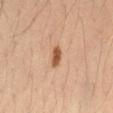workup: total-body-photography surveillance lesion; no biopsy | patient: male, aged around 35 | anatomic site: the lower back | imaging modality: 15 mm crop, total-body photography | size: ~3 mm (longest diameter) | illumination: cross-polarized | TBP lesion metrics: a lesion area of about 4 mm²; a lesion color around L≈50 a*≈21 b*≈33 in CIELAB, a lesion–skin lightness drop of about 12, and a lesion-to-skin contrast of about 9 (normalized; higher = more distinct).